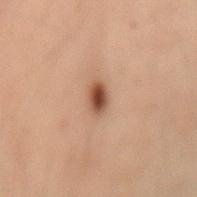Q: Was this lesion biopsied?
A: imaged on a skin check; not biopsied
Q: Illumination type?
A: cross-polarized
Q: What is the anatomic site?
A: the back
Q: How was this image acquired?
A: ~15 mm tile from a whole-body skin photo
Q: Automated lesion metrics?
A: a lesion color around L≈39 a*≈18 b*≈25 in CIELAB, roughly 13 lightness units darker than nearby skin, and a normalized lesion–skin contrast near 10.5; a border-irregularity index near 3/10, internal color variation of about 3 on a 0–10 scale, and radial color variation of about 1; a classifier nevus-likeness of about 100/100 and a lesion-detection confidence of about 100/100
Q: Patient demographics?
A: male, about 40 years old
Q: What is the lesion's diameter?
A: ~3 mm (longest diameter)